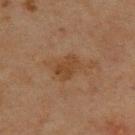<record>
  <biopsy_status>not biopsied; imaged during a skin examination</biopsy_status>
  <patient>
    <sex>male</sex>
    <age_approx>45</age_approx>
  </patient>
  <lesion_size>
    <long_diameter_mm_approx>3.0</long_diameter_mm_approx>
  </lesion_size>
  <lighting>cross-polarized</lighting>
  <site>upper back</site>
  <image>
    <source>total-body photography crop</source>
    <field_of_view_mm>15</field_of_view_mm>
  </image>
</record>